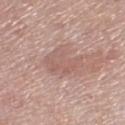Impression:
Part of a total-body skin-imaging series; this lesion was reviewed on a skin check and was not flagged for biopsy.
Acquisition and patient details:
This is a white-light tile. The lesion's longest dimension is about 4.5 mm. The lesion-visualizer software estimated an outline eccentricity of about 0.75 (0 = round, 1 = elongated). The analysis additionally found a lesion color around L≈58 a*≈20 b*≈24 in CIELAB, a lesion–skin lightness drop of about 7, and a normalized border contrast of about 4.5. The software also gave border irregularity of about 5 on a 0–10 scale and a within-lesion color-variation index near 2.5/10. It also reported a nevus-likeness score of about 0/100 and a detector confidence of about 95 out of 100 that the crop contains a lesion. A 15 mm close-up extracted from a 3D total-body photography capture. A female patient roughly 70 years of age. The lesion is located on the right lower leg.